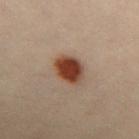Part of a total-body skin-imaging series; this lesion was reviewed on a skin check and was not flagged for biopsy. A female patient, about 30 years old. From the chest. The total-body-photography lesion software estimated an area of roughly 8.5 mm², a shape eccentricity near 0.6, and two-axis asymmetry of about 0.2. And it measured a mean CIELAB color near L≈32 a*≈19 b*≈25, a lesion–skin lightness drop of about 14, and a lesion-to-skin contrast of about 13 (normalized; higher = more distinct). The recorded lesion diameter is about 3.5 mm. A roughly 15 mm field-of-view crop from a total-body skin photograph. Imaged with cross-polarized lighting.